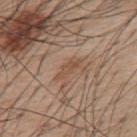Clinical impression: This lesion was catalogued during total-body skin photography and was not selected for biopsy. Background: The patient is a male in their mid-50s. This is a white-light tile. The lesion is on the upper back. A 15 mm crop from a total-body photograph taken for skin-cancer surveillance. Measured at roughly 3.5 mm in maximum diameter. Automated tile analysis of the lesion measured a footprint of about 3 mm², an eccentricity of roughly 0.95, and a shape-asymmetry score of about 0.3 (0 = symmetric). The analysis additionally found a lesion color around L≈50 a*≈18 b*≈30 in CIELAB, roughly 7 lightness units darker than nearby skin, and a lesion-to-skin contrast of about 5.5 (normalized; higher = more distinct). The analysis additionally found a within-lesion color-variation index near 0.5/10 and peripheral color asymmetry of about 0. And it measured an automated nevus-likeness rating near 5 out of 100 and lesion-presence confidence of about 90/100.